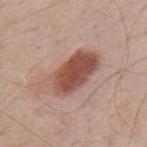{
  "biopsy_status": "not biopsied; imaged during a skin examination",
  "patient": {
    "sex": "male",
    "age_approx": 70
  },
  "site": "mid back",
  "lesion_size": {
    "long_diameter_mm_approx": 6.5
  },
  "image": {
    "source": "total-body photography crop",
    "field_of_view_mm": 15
  },
  "lighting": "white-light"
}